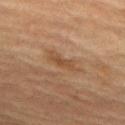Q: Was this lesion biopsied?
A: catalogued during a skin exam; not biopsied
Q: What lighting was used for the tile?
A: cross-polarized illumination
Q: What kind of image is this?
A: 15 mm crop, total-body photography
Q: Who is the patient?
A: male, in their mid- to late 70s
Q: Where on the body is the lesion?
A: the upper back